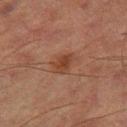No biopsy was performed on this lesion — it was imaged during a full skin examination and was not determined to be concerning.